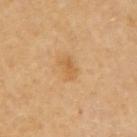notes: catalogued during a skin exam; not biopsied
size: about 2.5 mm
imaging modality: 15 mm crop, total-body photography
subject: female, aged around 70
site: the right upper arm